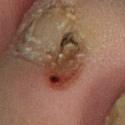Captured during whole-body skin photography for melanoma surveillance; the lesion was not biopsied.